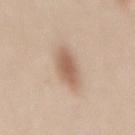Q: Is there a histopathology result?
A: no biopsy performed (imaged during a skin exam)
Q: How was this image acquired?
A: total-body-photography crop, ~15 mm field of view
Q: Lesion location?
A: the mid back
Q: What are the patient's age and sex?
A: female, roughly 30 years of age
Q: What lighting was used for the tile?
A: white-light
Q: How large is the lesion?
A: ≈4 mm